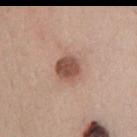Q: Was this lesion biopsied?
A: catalogued during a skin exam; not biopsied
Q: What is the lesion's diameter?
A: about 3 mm
Q: What are the patient's age and sex?
A: male, aged 38 to 42
Q: What kind of image is this?
A: ~15 mm crop, total-body skin-cancer survey
Q: What is the anatomic site?
A: the mid back
Q: Automated lesion metrics?
A: a footprint of about 7 mm², a shape eccentricity near 0.3, and a shape-asymmetry score of about 0.1 (0 = symmetric); a border-irregularity index near 1/10 and a color-variation rating of about 3/10; an automated nevus-likeness rating near 65 out of 100
Q: How was the tile lit?
A: white-light illumination